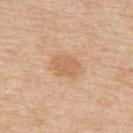Captured during whole-body skin photography for melanoma surveillance; the lesion was not biopsied.
On the back.
The patient is a male aged approximately 60.
Longest diameter approximately 3.5 mm.
The total-body-photography lesion software estimated a lesion area of about 8.5 mm² and two-axis asymmetry of about 0.15. It also reported a mean CIELAB color near L≈64 a*≈20 b*≈36 and about 8 CIELAB-L* units darker than the surrounding skin. The software also gave border irregularity of about 2 on a 0–10 scale, internal color variation of about 2 on a 0–10 scale, and a peripheral color-asymmetry measure near 0.5. And it measured a classifier nevus-likeness of about 10/100 and a lesion-detection confidence of about 100/100.
Captured under white-light illumination.
Cropped from a whole-body photographic skin survey; the tile spans about 15 mm.